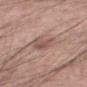follow-up: catalogued during a skin exam; not biopsied | site: the mid back | subject: male, approximately 55 years of age | image: total-body-photography crop, ~15 mm field of view.